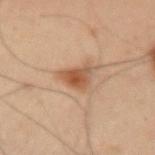lighting=cross-polarized illumination | image=15 mm crop, total-body photography | patient=male, aged around 55 | body site=the left upper arm.